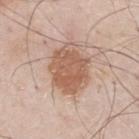notes = catalogued during a skin exam; not biopsied
acquisition = ~15 mm crop, total-body skin-cancer survey
subject = male, aged 78–82
lighting = white-light
location = the mid back